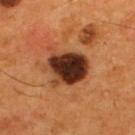This lesion was catalogued during total-body skin photography and was not selected for biopsy.
The lesion-visualizer software estimated a footprint of about 17 mm², an eccentricity of roughly 0.55, and a symmetry-axis asymmetry near 0.2. It also reported a detector confidence of about 100 out of 100 that the crop contains a lesion.
This is a cross-polarized tile.
The recorded lesion diameter is about 5 mm.
A male subject about 55 years old.
The lesion is on the back.
A lesion tile, about 15 mm wide, cut from a 3D total-body photograph.